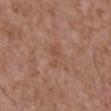<lesion>
<biopsy_status>not biopsied; imaged during a skin examination</biopsy_status>
<image>
  <source>total-body photography crop</source>
  <field_of_view_mm>15</field_of_view_mm>
</image>
<patient>
  <sex>male</sex>
  <age_approx>75</age_approx>
</patient>
<site>abdomen</site>
<automated_metrics>
  <area_mm2_approx>3.0</area_mm2_approx>
  <shape_asymmetry>0.35</shape_asymmetry>
  <cielab_L>50</cielab_L>
  <cielab_a>20</cielab_a>
  <cielab_b>30</cielab_b>
  <vs_skin_darker_L>6.0</vs_skin_darker_L>
  <vs_skin_contrast_norm>5.0</vs_skin_contrast_norm>
  <border_irregularity_0_10>4.0</border_irregularity_0_10>
</automated_metrics>
<lesion_size>
  <long_diameter_mm_approx>2.5</long_diameter_mm_approx>
</lesion_size>
<lighting>white-light</lighting>
</lesion>